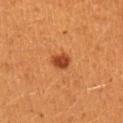| key | value |
|---|---|
| workup | no biopsy performed (imaged during a skin exam) |
| tile lighting | cross-polarized illumination |
| diameter | about 2.5 mm |
| patient | female, in their 30s |
| imaging modality | total-body-photography crop, ~15 mm field of view |
| anatomic site | the left upper arm |
| image-analysis metrics | an area of roughly 4.5 mm²; an average lesion color of about L≈43 a*≈29 b*≈39 (CIELAB), a lesion–skin lightness drop of about 13, and a normalized lesion–skin contrast near 9.5; a border-irregularity index near 2/10, internal color variation of about 2.5 on a 0–10 scale, and a peripheral color-asymmetry measure near 1; a nevus-likeness score of about 100/100 and lesion-presence confidence of about 100/100 |